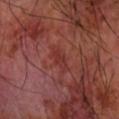workup: total-body-photography surveillance lesion; no biopsy
illumination: cross-polarized
site: the right forearm
subject: male, aged 68–72
diameter: ~3.5 mm (longest diameter)
acquisition: 15 mm crop, total-body photography